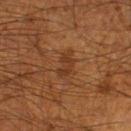Recorded during total-body skin imaging; not selected for excision or biopsy. This is a cross-polarized tile. About 3 mm across. A male patient in their 60s. Cropped from a total-body skin-imaging series; the visible field is about 15 mm. The lesion is located on the left thigh. Automated image analysis of the tile measured a footprint of about 4 mm², a shape eccentricity near 0.8, and a shape-asymmetry score of about 0.4 (0 = symmetric). The analysis additionally found an average lesion color of about L≈29 a*≈19 b*≈29 (CIELAB), roughly 6 lightness units darker than nearby skin, and a normalized border contrast of about 6. And it measured a color-variation rating of about 0.5/10.